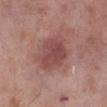| field | value |
|---|---|
| workup | imaged on a skin check; not biopsied |
| illumination | white-light |
| image-analysis metrics | roughly 10 lightness units darker than nearby skin and a normalized lesion–skin contrast near 7; a border-irregularity index near 2/10, internal color variation of about 3.5 on a 0–10 scale, and a peripheral color-asymmetry measure near 1 |
| subject | male, aged 68–72 |
| anatomic site | the right lower leg |
| acquisition | ~15 mm tile from a whole-body skin photo |